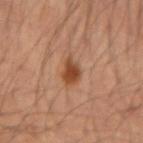The lesion was tiled from a total-body skin photograph and was not biopsied. A male patient, aged approximately 35. Cropped from a total-body skin-imaging series; the visible field is about 15 mm. The lesion is on the left forearm.